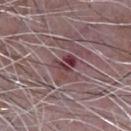The lesion was tiled from a total-body skin photograph and was not biopsied. This is a white-light tile. Longest diameter approximately 3 mm. A 15 mm crop from a total-body photograph taken for skin-cancer surveillance. Located on the front of the torso. The subject is a male aged 63 to 67.